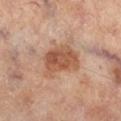A 15 mm close-up extracted from a 3D total-body photography capture. Measured at roughly 4.5 mm in maximum diameter. The lesion is on the right lower leg. The tile uses cross-polarized illumination. The lesion-visualizer software estimated an area of roughly 13 mm², an outline eccentricity of about 0.75 (0 = round, 1 = elongated), and a symmetry-axis asymmetry near 0.25. The software also gave a classifier nevus-likeness of about 50/100. A male patient about 65 years old.